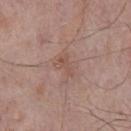Background: Located on the front of the torso. Approximately 3 mm at its widest. A male patient aged 73–77. A lesion tile, about 15 mm wide, cut from a 3D total-body photograph.